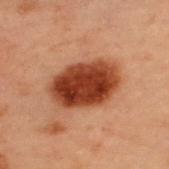biopsy status: no biopsy performed (imaged during a skin exam)
lesion diameter: ~6.5 mm (longest diameter)
imaging modality: ~15 mm tile from a whole-body skin photo
body site: the upper back
illumination: cross-polarized illumination
subject: male, aged 53 to 57